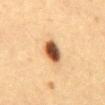biopsy_status: not biopsied; imaged during a skin examination
lighting: cross-polarized
image:
  source: total-body photography crop
  field_of_view_mm: 15
lesion_size:
  long_diameter_mm_approx: 3.5
automated_metrics:
  vs_skin_darker_L: 19.0
  vs_skin_contrast_norm: 13.5
  border_irregularity_0_10: 2.0
  color_variation_0_10: 8.0
  peripheral_color_asymmetry: 2.0
  nevus_likeness_0_100: 100
  lesion_detection_confidence_0_100: 100
site: abdomen
patient:
  sex: female
  age_approx: 40